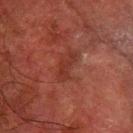<tbp_lesion>
  <biopsy_status>not biopsied; imaged during a skin examination</biopsy_status>
  <automated_metrics>
    <area_mm2_approx>5.0</area_mm2_approx>
    <eccentricity>0.95</eccentricity>
    <shape_asymmetry>0.3</shape_asymmetry>
    <cielab_L>27</cielab_L>
    <cielab_a>23</cielab_a>
    <cielab_b>23</cielab_b>
    <vs_skin_darker_L>5.0</vs_skin_darker_L>
    <vs_skin_contrast_norm>5.5</vs_skin_contrast_norm>
    <border_irregularity_0_10>4.0</border_irregularity_0_10>
    <color_variation_0_10>1.0</color_variation_0_10>
    <peripheral_color_asymmetry>0.0</peripheral_color_asymmetry>
  </automated_metrics>
  <lighting>cross-polarized</lighting>
  <site>right forearm</site>
  <image>
    <source>total-body photography crop</source>
    <field_of_view_mm>15</field_of_view_mm>
  </image>
  <patient>
    <sex>male</sex>
    <age_approx>80</age_approx>
  </patient>
  <lesion_size>
    <long_diameter_mm_approx>4.0</long_diameter_mm_approx>
  </lesion_size>
</tbp_lesion>